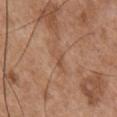Q: Was this lesion biopsied?
A: no biopsy performed (imaged during a skin exam)
Q: How was the tile lit?
A: white-light
Q: Patient demographics?
A: male, in their mid- to late 50s
Q: What kind of image is this?
A: ~15 mm tile from a whole-body skin photo
Q: What is the anatomic site?
A: the chest
Q: Lesion size?
A: about 3 mm
Q: What did automated image analysis measure?
A: a lesion area of about 4 mm², an outline eccentricity of about 0.9 (0 = round, 1 = elongated), and two-axis asymmetry of about 0.35; an average lesion color of about L≈52 a*≈20 b*≈32 (CIELAB), about 6 CIELAB-L* units darker than the surrounding skin, and a normalized border contrast of about 4.5; border irregularity of about 4 on a 0–10 scale and a peripheral color-asymmetry measure near 0.5